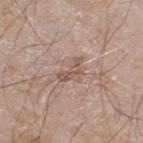notes: total-body-photography surveillance lesion; no biopsy | lesion diameter: ~3.5 mm (longest diameter) | subject: male, aged approximately 60 | body site: the right thigh | acquisition: 15 mm crop, total-body photography.